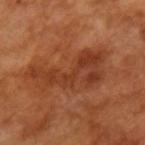Q: Was a biopsy performed?
A: imaged on a skin check; not biopsied
Q: Who is the patient?
A: male, in their mid- to late 60s
Q: How was this image acquired?
A: ~15 mm crop, total-body skin-cancer survey